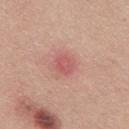  biopsy_status: not biopsied; imaged during a skin examination
  site: mid back
  lighting: white-light
  lesion_size:
    long_diameter_mm_approx: 2.5
  image:
    source: total-body photography crop
    field_of_view_mm: 15
  patient:
    sex: male
    age_approx: 25
  automated_metrics:
    color_variation_0_10: 3.0
    peripheral_color_asymmetry: 1.0
    nevus_likeness_0_100: 0